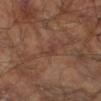Assessment: Recorded during total-body skin imaging; not selected for excision or biopsy. Image and clinical context: Automated image analysis of the tile measured two-axis asymmetry of about 0.5. It also reported an average lesion color of about L≈38 a*≈20 b*≈24 (CIELAB), roughly 6 lightness units darker than nearby skin, and a lesion-to-skin contrast of about 5.5 (normalized; higher = more distinct). The analysis additionally found an automated nevus-likeness rating near 0 out of 100 and a detector confidence of about 60 out of 100 that the crop contains a lesion. On the left forearm. A male patient in their mid- to late 60s. This is a cross-polarized tile. A 15 mm close-up tile from a total-body photography series done for melanoma screening.